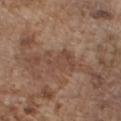follow-up: imaged on a skin check; not biopsied | illumination: white-light | body site: the chest | acquisition: 15 mm crop, total-body photography | patient: male, in their mid- to late 70s | size: ≈4 mm | automated lesion analysis: an eccentricity of roughly 0.8 and a symmetry-axis asymmetry near 0.45; a border-irregularity rating of about 5.5/10 and internal color variation of about 2 on a 0–10 scale.